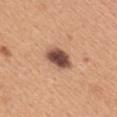Notes:
- workup · no biopsy performed (imaged during a skin exam)
- anatomic site · the upper back
- tile lighting · white-light illumination
- size · about 4 mm
- automated lesion analysis · a border-irregularity index near 2/10, a color-variation rating of about 5/10, and peripheral color asymmetry of about 1.5; an automated nevus-likeness rating near 75 out of 100
- image · total-body-photography crop, ~15 mm field of view
- patient · female, approximately 30 years of age